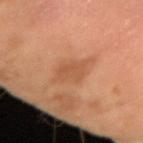| feature | finding |
|---|---|
| biopsy status | no biopsy performed (imaged during a skin exam) |
| acquisition | 15 mm crop, total-body photography |
| lesion size | ≈4.5 mm |
| illumination | cross-polarized illumination |
| patient | male, aged around 65 |